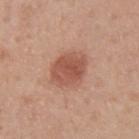{"biopsy_status": "not biopsied; imaged during a skin examination", "lesion_size": {"long_diameter_mm_approx": 4.5}, "site": "upper back", "image": {"source": "total-body photography crop", "field_of_view_mm": 15}, "automated_metrics": {"vs_skin_darker_L": 11.0, "border_irregularity_0_10": 1.5, "peripheral_color_asymmetry": 1.0, "nevus_likeness_0_100": 100, "lesion_detection_confidence_0_100": 100}, "patient": {"sex": "male", "age_approx": 40}, "lighting": "white-light"}